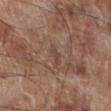Recorded during total-body skin imaging; not selected for excision or biopsy. A 15 mm crop from a total-body photograph taken for skin-cancer surveillance. A male subject, aged approximately 70. The lesion is on the right lower leg.